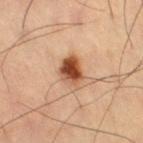The lesion was tiled from a total-body skin photograph and was not biopsied.
The lesion is located on the leg.
A 15 mm close-up extracted from a 3D total-body photography capture.
About 3.5 mm across.
The tile uses cross-polarized illumination.
The total-body-photography lesion software estimated a within-lesion color-variation index near 8.5/10 and radial color variation of about 2.5. It also reported a classifier nevus-likeness of about 100/100 and a lesion-detection confidence of about 100/100.
A male patient, approximately 60 years of age.